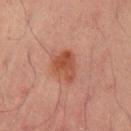Q: Lesion size?
A: ≈3.5 mm
Q: What did automated image analysis measure?
A: a footprint of about 7.5 mm², a shape eccentricity near 0.65, and a shape-asymmetry score of about 0.35 (0 = symmetric); an average lesion color of about L≈37 a*≈22 b*≈26 (CIELAB), about 8 CIELAB-L* units darker than the surrounding skin, and a normalized lesion–skin contrast near 8; a border-irregularity index near 3/10, a color-variation rating of about 4/10, and a peripheral color-asymmetry measure near 1.5; a nevus-likeness score of about 45/100 and a lesion-detection confidence of about 100/100
Q: What lighting was used for the tile?
A: cross-polarized illumination
Q: What is the imaging modality?
A: total-body-photography crop, ~15 mm field of view
Q: Who is the patient?
A: male, in their 50s
Q: Where on the body is the lesion?
A: the arm